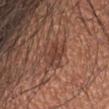{
  "automated_metrics": {
    "cielab_L": 41,
    "cielab_a": 22,
    "cielab_b": 26,
    "vs_skin_darker_L": 7.0
  },
  "image": {
    "source": "total-body photography crop",
    "field_of_view_mm": 15
  },
  "site": "head or neck",
  "lesion_size": {
    "long_diameter_mm_approx": 4.0
  },
  "patient": {
    "sex": "male",
    "age_approx": 45
  }
}